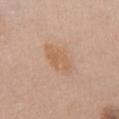Cropped from a total-body skin-imaging series; the visible field is about 15 mm. The subject is a female about 60 years old. Captured under white-light illumination. Located on the chest.